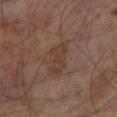Assessment: The lesion was tiled from a total-body skin photograph and was not biopsied. Context: Measured at roughly 4 mm in maximum diameter. Automated image analysis of the tile measured a lesion area of about 8 mm², an outline eccentricity of about 0.85 (0 = round, 1 = elongated), and two-axis asymmetry of about 0.4. And it measured an automated nevus-likeness rating near 0 out of 100 and a lesion-detection confidence of about 100/100. From the leg. A male subject, approximately 65 years of age. Captured under cross-polarized illumination. A 15 mm close-up extracted from a 3D total-body photography capture.